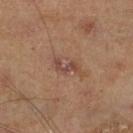follow-up=total-body-photography surveillance lesion; no biopsy | subject=male, aged approximately 45 | image source=~15 mm crop, total-body skin-cancer survey | site=the right lower leg.